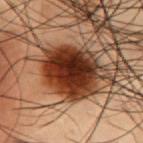notes = imaged on a skin check; not biopsied | lighting = cross-polarized | subject = male, aged approximately 55 | lesion diameter = ~6.5 mm (longest diameter) | anatomic site = the chest | image-analysis metrics = an average lesion color of about L≈27 a*≈21 b*≈27 (CIELAB), about 18 CIELAB-L* units darker than the surrounding skin, and a normalized lesion–skin contrast near 16.5; a border-irregularity rating of about 1.5/10, a within-lesion color-variation index near 8/10, and peripheral color asymmetry of about 2 | imaging modality = ~15 mm crop, total-body skin-cancer survey.